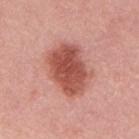workup: no biopsy performed (imaged during a skin exam).